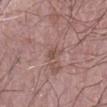Q: Is there a histopathology result?
A: no biopsy performed (imaged during a skin exam)
Q: What is the imaging modality?
A: ~15 mm crop, total-body skin-cancer survey
Q: What is the anatomic site?
A: the arm
Q: How was the tile lit?
A: white-light
Q: Who is the patient?
A: male, about 60 years old